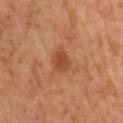The lesion was photographed on a routine skin check and not biopsied; there is no pathology result.
A lesion tile, about 15 mm wide, cut from a 3D total-body photograph.
Captured under cross-polarized illumination.
The lesion's longest dimension is about 3 mm.
The subject is a male in their mid- to late 60s.